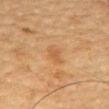Q: Is there a histopathology result?
A: total-body-photography surveillance lesion; no biopsy
Q: Who is the patient?
A: male, roughly 85 years of age or older
Q: Lesion size?
A: ~2.5 mm (longest diameter)
Q: Where on the body is the lesion?
A: the chest
Q: How was this image acquired?
A: total-body-photography crop, ~15 mm field of view
Q: Illumination type?
A: cross-polarized illumination